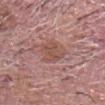Imaged during a routine full-body skin examination; the lesion was not biopsied and no histopathology is available.